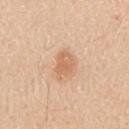Case summary:
* lesion size · about 3.5 mm
* site · the right upper arm
* subject · male, about 30 years old
* image source · total-body-photography crop, ~15 mm field of view